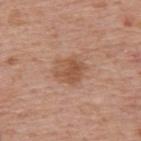This lesion was catalogued during total-body skin photography and was not selected for biopsy.
The lesion is on the upper back.
The subject is a male aged approximately 75.
Cropped from a whole-body photographic skin survey; the tile spans about 15 mm.